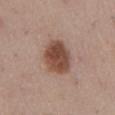Notes:
• follow-up — total-body-photography surveillance lesion; no biopsy
• subject — male, aged around 60
• acquisition — ~15 mm crop, total-body skin-cancer survey
• anatomic site — the chest